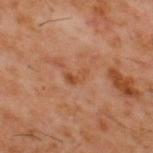Findings:
- biopsy status: total-body-photography surveillance lesion; no biopsy
- acquisition: ~15 mm crop, total-body skin-cancer survey
- image-analysis metrics: a footprint of about 3 mm², a shape eccentricity near 0.85, and two-axis asymmetry of about 0.45; border irregularity of about 4.5 on a 0–10 scale, internal color variation of about 0 on a 0–10 scale, and radial color variation of about 0; an automated nevus-likeness rating near 0 out of 100 and lesion-presence confidence of about 100/100
- patient: male, approximately 60 years of age
- tile lighting: cross-polarized
- anatomic site: the upper back